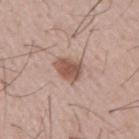Case summary:
- workup — total-body-photography surveillance lesion; no biopsy
- subject — male, aged 53–57
- image — ~15 mm tile from a whole-body skin photo